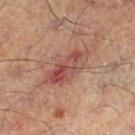{"biopsy_status": "not biopsied; imaged during a skin examination", "patient": {"sex": "male", "age_approx": 65}, "image": {"source": "total-body photography crop", "field_of_view_mm": 15}, "lesion_size": {"long_diameter_mm_approx": 6.5}, "site": "left lower leg"}